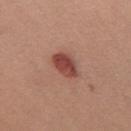Clinical impression: Part of a total-body skin-imaging series; this lesion was reviewed on a skin check and was not flagged for biopsy. Background: Measured at roughly 4 mm in maximum diameter. Captured under white-light illumination. A female patient aged approximately 35. The lesion is on the back. The lesion-visualizer software estimated a footprint of about 7.5 mm², an outline eccentricity of about 0.75 (0 = round, 1 = elongated), and two-axis asymmetry of about 0.2. This image is a 15 mm lesion crop taken from a total-body photograph.